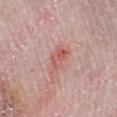follow-up = catalogued during a skin exam; not biopsied | subject = male, about 65 years old | lighting = white-light illumination | lesion diameter = about 3 mm | imaging modality = total-body-photography crop, ~15 mm field of view | body site = the left lower leg.